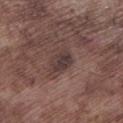Q: How large is the lesion?
A: about 3 mm
Q: Who is the patient?
A: male, approximately 75 years of age
Q: Automated lesion metrics?
A: a footprint of about 5 mm², an eccentricity of roughly 0.65, and a symmetry-axis asymmetry near 0.15; roughly 8 lightness units darker than nearby skin and a normalized border contrast of about 8
Q: How was this image acquired?
A: ~15 mm tile from a whole-body skin photo
Q: Lesion location?
A: the leg